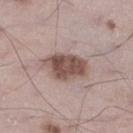Q: Is there a histopathology result?
A: total-body-photography surveillance lesion; no biopsy
Q: Who is the patient?
A: male, aged approximately 70
Q: Illumination type?
A: white-light
Q: Lesion location?
A: the left lower leg
Q: How large is the lesion?
A: ≈6 mm
Q: What is the imaging modality?
A: ~15 mm crop, total-body skin-cancer survey